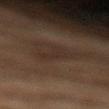Recorded during total-body skin imaging; not selected for excision or biopsy. This image is a 15 mm lesion crop taken from a total-body photograph. The lesion is on the left lower leg. The subject is a male about 45 years old.